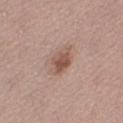biopsy_status: not biopsied; imaged during a skin examination
patient:
  sex: female
  age_approx: 50
site: left thigh
image:
  source: total-body photography crop
  field_of_view_mm: 15